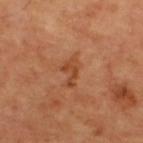follow-up: imaged on a skin check; not biopsied | site: the upper back | subject: male, roughly 60 years of age | image source: total-body-photography crop, ~15 mm field of view | TBP lesion metrics: an area of roughly 4 mm² and an outline eccentricity of about 0.85 (0 = round, 1 = elongated).